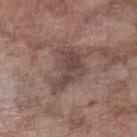The lesion was tiled from a total-body skin photograph and was not biopsied. A male subject, aged around 75. Cropped from a whole-body photographic skin survey; the tile spans about 15 mm. Imaged with white-light lighting. On the leg.